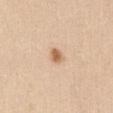<record>
  <biopsy_status>not biopsied; imaged during a skin examination</biopsy_status>
  <lesion_size>
    <long_diameter_mm_approx>2.0</long_diameter_mm_approx>
  </lesion_size>
  <automated_metrics>
    <area_mm2_approx>3.0</area_mm2_approx>
    <eccentricity>0.45</eccentricity>
    <shape_asymmetry>0.15</shape_asymmetry>
  </automated_metrics>
  <lighting>white-light</lighting>
  <site>abdomen</site>
  <patient>
    <sex>female</sex>
    <age_approx>20</age_approx>
  </patient>
  <image>
    <source>total-body photography crop</source>
    <field_of_view_mm>15</field_of_view_mm>
  </image>
</record>